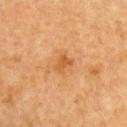Image and clinical context:
A close-up tile cropped from a whole-body skin photograph, about 15 mm across. This is a cross-polarized tile. The lesion's longest dimension is about 2.5 mm. A male subject aged 63 to 67. From the right upper arm.